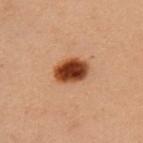Part of a total-body skin-imaging series; this lesion was reviewed on a skin check and was not flagged for biopsy.
Imaged with cross-polarized lighting.
A female patient, aged approximately 50.
A lesion tile, about 15 mm wide, cut from a 3D total-body photograph.
Automated tile analysis of the lesion measured a classifier nevus-likeness of about 100/100 and a detector confidence of about 100 out of 100 that the crop contains a lesion.
Located on the chest.
Measured at roughly 3.5 mm in maximum diameter.